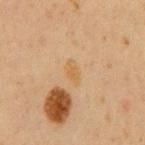biopsy status = catalogued during a skin exam; not biopsied | patient = male, about 60 years old | acquisition = total-body-photography crop, ~15 mm field of view | size = ~3 mm (longest diameter) | location = the mid back | tile lighting = cross-polarized illumination.